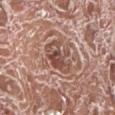Clinical impression:
No biopsy was performed on this lesion — it was imaged during a full skin examination and was not determined to be concerning.
Acquisition and patient details:
The patient is a male in their mid- to late 70s. This image is a 15 mm lesion crop taken from a total-body photograph. Approximately 4 mm at its widest. Located on the right lower leg. The lesion-visualizer software estimated a shape eccentricity near 0.8 and two-axis asymmetry of about 0.4. It also reported a mean CIELAB color near L≈49 a*≈21 b*≈25 and a lesion-to-skin contrast of about 8 (normalized; higher = more distinct). And it measured a classifier nevus-likeness of about 0/100.